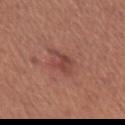Q: Was this lesion biopsied?
A: imaged on a skin check; not biopsied
Q: Illumination type?
A: white-light illumination
Q: Who is the patient?
A: male, aged approximately 45
Q: What is the imaging modality?
A: 15 mm crop, total-body photography
Q: What is the lesion's diameter?
A: ≈3 mm
Q: Lesion location?
A: the left upper arm